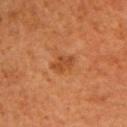No biopsy was performed on this lesion — it was imaged during a full skin examination and was not determined to be concerning.
The patient is a male roughly 60 years of age.
The lesion-visualizer software estimated an area of roughly 4.5 mm², a shape eccentricity near 0.85, and a shape-asymmetry score of about 0.3 (0 = symmetric). It also reported an average lesion color of about L≈39 a*≈23 b*≈34 (CIELAB).
A close-up tile cropped from a whole-body skin photograph, about 15 mm across.
Imaged with cross-polarized lighting.
The lesion is located on the left upper arm.